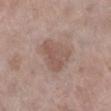Imaged during a routine full-body skin examination; the lesion was not biopsied and no histopathology is available. The total-body-photography lesion software estimated an area of roughly 12 mm², an outline eccentricity of about 0.55 (0 = round, 1 = elongated), and a symmetry-axis asymmetry near 0.35. A female subject, about 75 years old. A lesion tile, about 15 mm wide, cut from a 3D total-body photograph. The lesion is on the left lower leg.